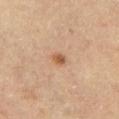Recorded during total-body skin imaging; not selected for excision or biopsy.
Longest diameter approximately 1.5 mm.
The lesion-visualizer software estimated a shape eccentricity near 0.65 and a symmetry-axis asymmetry near 0.3. The analysis additionally found an automated nevus-likeness rating near 90 out of 100 and a lesion-detection confidence of about 100/100.
From the right thigh.
The tile uses cross-polarized illumination.
The subject is a female in their mid-60s.
A 15 mm crop from a total-body photograph taken for skin-cancer surveillance.